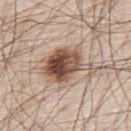Assessment: No biopsy was performed on this lesion — it was imaged during a full skin examination and was not determined to be concerning. Background: A region of skin cropped from a whole-body photographic capture, roughly 15 mm wide. The tile uses white-light illumination. A male patient, aged around 80. The recorded lesion diameter is about 6.5 mm. Located on the upper back. The total-body-photography lesion software estimated a lesion color around L≈51 a*≈17 b*≈27 in CIELAB, roughly 17 lightness units darker than nearby skin, and a normalized border contrast of about 11.5. It also reported border irregularity of about 4.5 on a 0–10 scale and internal color variation of about 9.5 on a 0–10 scale. It also reported lesion-presence confidence of about 100/100.